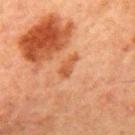workup = no biopsy performed (imaged during a skin exam)
patient = male, aged approximately 65
body site = the chest
image = 15 mm crop, total-body photography